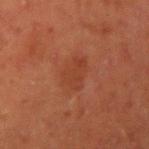| field | value |
|---|---|
| patient | male, aged approximately 45 |
| image | ~15 mm crop, total-body skin-cancer survey |
| site | the right upper arm |